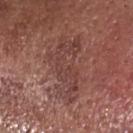Clinical impression: Imaged during a routine full-body skin examination; the lesion was not biopsied and no histopathology is available. Acquisition and patient details: Measured at roughly 7.5 mm in maximum diameter. Located on the head or neck. A 15 mm close-up extracted from a 3D total-body photography capture. The subject is a male aged 63–67.